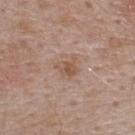  biopsy_status: not biopsied; imaged during a skin examination
  site: back
  image:
    source: total-body photography crop
    field_of_view_mm: 15
  lesion_size:
    long_diameter_mm_approx: 3.0
  patient:
    sex: male
    age_approx: 55
  lighting: white-light
  automated_metrics:
    eccentricity: 0.55
    border_irregularity_0_10: 4.5
    color_variation_0_10: 3.5